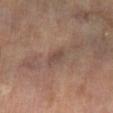Q: Was a biopsy performed?
A: total-body-photography surveillance lesion; no biopsy
Q: How was this image acquired?
A: ~15 mm crop, total-body skin-cancer survey
Q: What is the anatomic site?
A: the right leg
Q: What are the patient's age and sex?
A: female, aged approximately 65